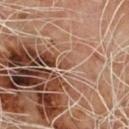Clinical summary: Automated image analysis of the tile measured border irregularity of about 2.5 on a 0–10 scale, internal color variation of about 0 on a 0–10 scale, and peripheral color asymmetry of about 0. It also reported an automated nevus-likeness rating near 0 out of 100. A 15 mm close-up extracted from a 3D total-body photography capture. The subject is a male roughly 65 years of age. This is a cross-polarized tile. The recorded lesion diameter is about 1.5 mm. The lesion is located on the chest.